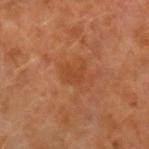image source: total-body-photography crop, ~15 mm field of view; patient: male, approximately 60 years of age; lesion diameter: ≈2.5 mm; location: the left forearm; illumination: cross-polarized.